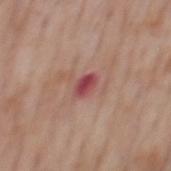notes: no biopsy performed (imaged during a skin exam)
image-analysis metrics: an area of roughly 4 mm², an outline eccentricity of about 0.75 (0 = round, 1 = elongated), and a symmetry-axis asymmetry near 0.3; a border-irregularity rating of about 2.5/10, internal color variation of about 3 on a 0–10 scale, and peripheral color asymmetry of about 1; a classifier nevus-likeness of about 0/100 and a detector confidence of about 100 out of 100 that the crop contains a lesion
tile lighting: white-light
diameter: ≈2.5 mm
patient: male, aged around 75
image: total-body-photography crop, ~15 mm field of view
location: the mid back